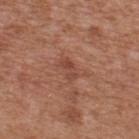Assessment:
This lesion was catalogued during total-body skin photography and was not selected for biopsy.
Image and clinical context:
Approximately 2.5 mm at its widest. A male subject aged 63–67. Cropped from a total-body skin-imaging series; the visible field is about 15 mm. The lesion is on the back. The lesion-visualizer software estimated a lesion color around L≈45 a*≈25 b*≈29 in CIELAB, roughly 8 lightness units darker than nearby skin, and a lesion-to-skin contrast of about 6 (normalized; higher = more distinct).